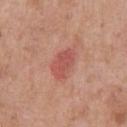{"biopsy_status": "not biopsied; imaged during a skin examination", "site": "chest", "lesion_size": {"long_diameter_mm_approx": 4.0}, "image": {"source": "total-body photography crop", "field_of_view_mm": 15}, "patient": {"sex": "male", "age_approx": 80}, "lighting": "white-light"}